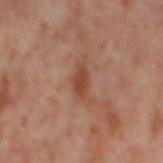No biopsy was performed on this lesion — it was imaged during a full skin examination and was not determined to be concerning. A female patient aged 53 to 57. A region of skin cropped from a whole-body photographic capture, roughly 15 mm wide. The lesion is on the left thigh.